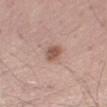Captured during whole-body skin photography for melanoma surveillance; the lesion was not biopsied.
This is a white-light tile.
Measured at roughly 3 mm in maximum diameter.
Located on the leg.
A lesion tile, about 15 mm wide, cut from a 3D total-body photograph.
A male patient, in their mid-50s.